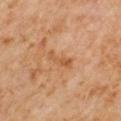<tbp_lesion>
  <biopsy_status>not biopsied; imaged during a skin examination</biopsy_status>
  <automated_metrics>
    <area_mm2_approx>4.5</area_mm2_approx>
    <eccentricity>0.9</eccentricity>
    <shape_asymmetry>0.35</shape_asymmetry>
    <nevus_likeness_0_100>0</nevus_likeness_0_100>
    <lesion_detection_confidence_0_100>100</lesion_detection_confidence_0_100>
  </automated_metrics>
  <patient>
    <sex>male</sex>
    <age_approx>60</age_approx>
  </patient>
  <image>
    <source>total-body photography crop</source>
    <field_of_view_mm>15</field_of_view_mm>
  </image>
  <lesion_size>
    <long_diameter_mm_approx>3.5</long_diameter_mm_approx>
  </lesion_size>
  <site>right upper arm</site>
  <lighting>cross-polarized</lighting>
</tbp_lesion>